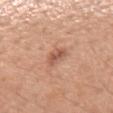The lesion was photographed on a routine skin check and not biopsied; there is no pathology result.
Located on the right forearm.
A female patient, aged around 45.
A lesion tile, about 15 mm wide, cut from a 3D total-body photograph.